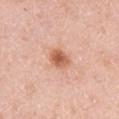Impression:
Part of a total-body skin-imaging series; this lesion was reviewed on a skin check and was not flagged for biopsy.
Context:
A female subject roughly 50 years of age. A roughly 15 mm field-of-view crop from a total-body skin photograph. From the left upper arm.